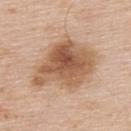Case summary:
* follow-up: total-body-photography surveillance lesion; no biopsy
* illumination: white-light illumination
* TBP lesion metrics: a mean CIELAB color near L≈58 a*≈19 b*≈32 and about 13 CIELAB-L* units darker than the surrounding skin; internal color variation of about 7 on a 0–10 scale and peripheral color asymmetry of about 2.5; a nevus-likeness score of about 60/100 and a detector confidence of about 100 out of 100 that the crop contains a lesion
* size: ≈8.5 mm
* image source: ~15 mm crop, total-body skin-cancer survey
* location: the upper back
* subject: male, aged approximately 60